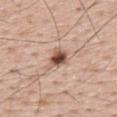The lesion was photographed on a routine skin check and not biopsied; there is no pathology result. A male subject approximately 65 years of age. A 15 mm crop from a total-body photograph taken for skin-cancer surveillance. Captured under white-light illumination. From the back.